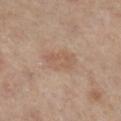Findings:
* biopsy status — imaged on a skin check; not biopsied
* image source — total-body-photography crop, ~15 mm field of view
* subject — female, aged around 60
* automated metrics — an automated nevus-likeness rating near 0 out of 100
* anatomic site — the left leg